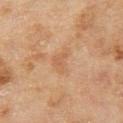Q: What kind of image is this?
A: ~15 mm tile from a whole-body skin photo
Q: What is the lesion's diameter?
A: about 3 mm
Q: Lesion location?
A: the left thigh
Q: Who is the patient?
A: female, approximately 60 years of age
Q: Illumination type?
A: cross-polarized illumination